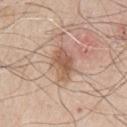workup=imaged on a skin check; not biopsied | image source=total-body-photography crop, ~15 mm field of view | subject=male, aged approximately 65 | lighting=white-light illumination | automated lesion analysis=a lesion area of about 8.5 mm², an eccentricity of roughly 0.8, and a symmetry-axis asymmetry near 0.2; roughly 11 lightness units darker than nearby skin and a normalized border contrast of about 7; a nevus-likeness score of about 40/100 | site=the chest.